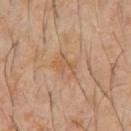workup: catalogued during a skin exam; not biopsied | subject: male, approximately 60 years of age | anatomic site: the chest | illumination: cross-polarized illumination | image: ~15 mm crop, total-body skin-cancer survey | lesion size: ≈3 mm.